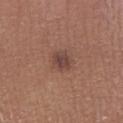Impression: The lesion was photographed on a routine skin check and not biopsied; there is no pathology result. Acquisition and patient details: A male patient aged 53–57. Cropped from a total-body skin-imaging series; the visible field is about 15 mm. On the right lower leg. An algorithmic analysis of the crop reported a lesion color around L≈43 a*≈20 b*≈23 in CIELAB, a lesion–skin lightness drop of about 9, and a normalized lesion–skin contrast near 8. The analysis additionally found a border-irregularity rating of about 2/10 and peripheral color asymmetry of about 1. The software also gave a classifier nevus-likeness of about 65/100 and a detector confidence of about 100 out of 100 that the crop contains a lesion. The tile uses white-light illumination.